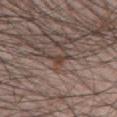Impression:
Recorded during total-body skin imaging; not selected for excision or biopsy.
Acquisition and patient details:
On the chest. The total-body-photography lesion software estimated roughly 8 lightness units darker than nearby skin and a normalized lesion–skin contrast near 7.5. It also reported an automated nevus-likeness rating near 5 out of 100 and a detector confidence of about 80 out of 100 that the crop contains a lesion. This is a white-light tile. A close-up tile cropped from a whole-body skin photograph, about 15 mm across. A male patient, about 40 years old. Longest diameter approximately 3 mm.